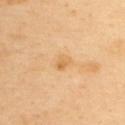biopsy status: imaged on a skin check; not biopsied | site: the upper back | image: ~15 mm crop, total-body skin-cancer survey | patient: male, aged 38–42.